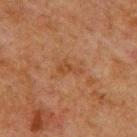Recorded during total-body skin imaging; not selected for excision or biopsy. On the upper back. A roughly 15 mm field-of-view crop from a total-body skin photograph. An algorithmic analysis of the crop reported a classifier nevus-likeness of about 0/100 and lesion-presence confidence of about 100/100. About 3 mm across. The patient is a male aged 58 to 62.